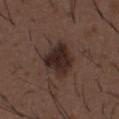biopsy status = total-body-photography surveillance lesion; no biopsy
anatomic site = the abdomen
diameter = about 5 mm
patient = male, aged 48 to 52
tile lighting = white-light
image = 15 mm crop, total-body photography